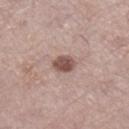{"biopsy_status": "not biopsied; imaged during a skin examination", "lesion_size": {"long_diameter_mm_approx": 2.5}, "patient": {"sex": "female", "age_approx": 65}, "site": "right lower leg", "image": {"source": "total-body photography crop", "field_of_view_mm": 15}, "lighting": "white-light", "automated_metrics": {"area_mm2_approx": 5.0, "eccentricity": 0.45, "shape_asymmetry": 0.15, "cielab_L": 51, "cielab_a": 18, "cielab_b": 22, "vs_skin_darker_L": 14.0, "border_irregularity_0_10": 1.5, "peripheral_color_asymmetry": 1.0, "nevus_likeness_0_100": 90}}